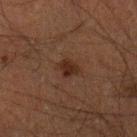  biopsy_status: not biopsied; imaged during a skin examination
  lighting: cross-polarized
  site: right lower leg
  image:
    source: total-body photography crop
    field_of_view_mm: 15
  automated_metrics:
    area_mm2_approx: 4.5
    eccentricity: 0.7
    shape_asymmetry: 0.3
    nevus_likeness_0_100: 90
    lesion_detection_confidence_0_100: 100
  patient:
    sex: male
    age_approx: 50
  lesion_size:
    long_diameter_mm_approx: 2.5